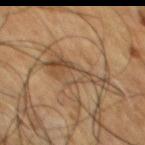follow-up: imaged on a skin check; not biopsied
site: the chest
patient: male, aged around 55
tile lighting: cross-polarized illumination
acquisition: total-body-photography crop, ~15 mm field of view
lesion size: ≈7 mm